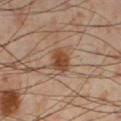biopsy status = no biopsy performed (imaged during a skin exam) | lighting = cross-polarized | image = 15 mm crop, total-body photography | image-analysis metrics = a shape eccentricity near 0.55 and a symmetry-axis asymmetry near 0.2; about 12 CIELAB-L* units darker than the surrounding skin; a border-irregularity rating of about 2/10, internal color variation of about 3 on a 0–10 scale, and radial color variation of about 1; a nevus-likeness score of about 95/100 | subject = male, in their mid- to late 50s | location = the left lower leg | lesion diameter = ≈2.5 mm.